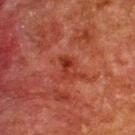Case summary:
– notes — total-body-photography surveillance lesion; no biopsy
– subject — male, aged around 60
– TBP lesion metrics — a lesion color around L≈29 a*≈28 b*≈27 in CIELAB and roughly 6 lightness units darker than nearby skin
– image source — total-body-photography crop, ~15 mm field of view
– lighting — cross-polarized illumination
– lesion size — ~2.5 mm (longest diameter)
– site — the upper back